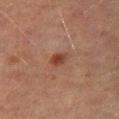Q: Was a biopsy performed?
A: imaged on a skin check; not biopsied
Q: Where on the body is the lesion?
A: the left thigh
Q: How was this image acquired?
A: ~15 mm crop, total-body skin-cancer survey
Q: Illumination type?
A: cross-polarized illumination
Q: What is the lesion's diameter?
A: ~2.5 mm (longest diameter)
Q: What are the patient's age and sex?
A: male, aged 68–72